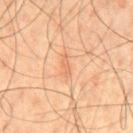  site: chest
  lighting: cross-polarized
  image:
    source: total-body photography crop
    field_of_view_mm: 15
  automated_metrics:
    cielab_L: 65
    cielab_a: 25
    cielab_b: 37
    vs_skin_darker_L: 7.0
    vs_skin_contrast_norm: 4.5
    border_irregularity_0_10: 3.5
    peripheral_color_asymmetry: 0.0
    nevus_likeness_0_100: 0
    lesion_detection_confidence_0_100: 100
  patient:
    sex: male
    age_approx: 45
  lesion_size:
    long_diameter_mm_approx: 3.0